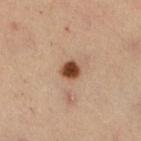Part of a total-body skin-imaging series; this lesion was reviewed on a skin check and was not flagged for biopsy. The recorded lesion diameter is about 2 mm. On the right lower leg. Automated image analysis of the tile measured an eccentricity of roughly 0.3 and a symmetry-axis asymmetry near 0.25. And it measured a lesion color around L≈45 a*≈22 b*≈32 in CIELAB, a lesion–skin lightness drop of about 18, and a normalized lesion–skin contrast near 13. This is a cross-polarized tile. A region of skin cropped from a whole-body photographic capture, roughly 15 mm wide. A female subject, about 40 years old.